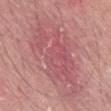Imaged during a routine full-body skin examination; the lesion was not biopsied and no histopathology is available. A male patient, aged around 40. Approximately 13 mm at its widest. This image is a 15 mm lesion crop taken from a total-body photograph. The tile uses white-light illumination. The lesion is on the abdomen.